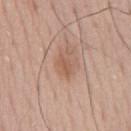Captured during whole-body skin photography for melanoma surveillance; the lesion was not biopsied. A lesion tile, about 15 mm wide, cut from a 3D total-body photograph. A male patient aged 73–77. The lesion is on the chest.